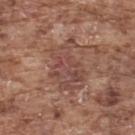No biopsy was performed on this lesion — it was imaged during a full skin examination and was not determined to be concerning.
Longest diameter approximately 6 mm.
A male subject, in their mid- to late 70s.
A 15 mm close-up tile from a total-body photography series done for melanoma screening.
Located on the upper back.